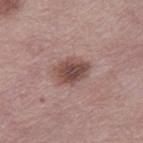No biopsy was performed on this lesion — it was imaged during a full skin examination and was not determined to be concerning.
The lesion is on the leg.
A 15 mm close-up tile from a total-body photography series done for melanoma screening.
A female patient, aged 63 to 67.